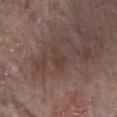Part of a total-body skin-imaging series; this lesion was reviewed on a skin check and was not flagged for biopsy. The lesion-visualizer software estimated a footprint of about 3.5 mm², a shape eccentricity near 0.7, and a symmetry-axis asymmetry near 0.35. It also reported border irregularity of about 3.5 on a 0–10 scale, internal color variation of about 1.5 on a 0–10 scale, and radial color variation of about 0.5. And it measured a nevus-likeness score of about 0/100. A region of skin cropped from a whole-body photographic capture, roughly 15 mm wide. The lesion is located on the right leg. A female subject, aged 78 to 82. The lesion's longest dimension is about 2.5 mm. Captured under cross-polarized illumination.